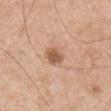Recorded during total-body skin imaging; not selected for excision or biopsy.
Cropped from a total-body skin-imaging series; the visible field is about 15 mm.
The lesion is on the right upper arm.
A male subject, aged 53 to 57.